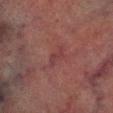The lesion is located on the right lower leg.
A male subject aged around 75.
The lesion-visualizer software estimated a lesion–skin lightness drop of about 5 and a lesion-to-skin contrast of about 5 (normalized; higher = more distinct). And it measured a lesion-detection confidence of about 80/100.
Longest diameter approximately 3 mm.
Cropped from a whole-body photographic skin survey; the tile spans about 15 mm.